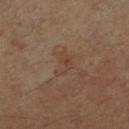Clinical impression: No biopsy was performed on this lesion — it was imaged during a full skin examination and was not determined to be concerning. Image and clinical context: A 15 mm crop from a total-body photograph taken for skin-cancer surveillance. Located on the arm. A male patient, aged 53 to 57.